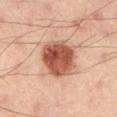biopsy_status: not biopsied; imaged during a skin examination
patient:
  sex: male
  age_approx: 40
site: left lower leg
lighting: cross-polarized
image:
  source: total-body photography crop
  field_of_view_mm: 15